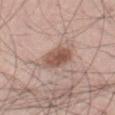Q: Was this lesion biopsied?
A: no biopsy performed (imaged during a skin exam)
Q: How was this image acquired?
A: total-body-photography crop, ~15 mm field of view
Q: Illumination type?
A: white-light illumination
Q: Patient demographics?
A: male, in their 30s
Q: What is the lesion's diameter?
A: ≈4 mm
Q: Lesion location?
A: the right thigh